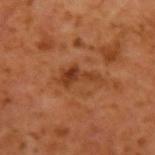Q: Was this lesion biopsied?
A: total-body-photography surveillance lesion; no biopsy
Q: Illumination type?
A: cross-polarized
Q: Lesion location?
A: the arm
Q: Lesion size?
A: ~5 mm (longest diameter)
Q: Who is the patient?
A: male, aged 58 to 62
Q: What is the imaging modality?
A: ~15 mm crop, total-body skin-cancer survey
Q: What did automated image analysis measure?
A: a lesion area of about 6.5 mm², a shape eccentricity near 0.9, and a symmetry-axis asymmetry near 0.6; an automated nevus-likeness rating near 0 out of 100 and a detector confidence of about 100 out of 100 that the crop contains a lesion